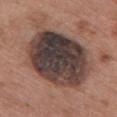Clinical summary:
Cropped from a whole-body photographic skin survey; the tile spans about 15 mm. The lesion is on the chest. A female subject aged 58–62.